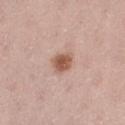notes: no biopsy performed (imaged during a skin exam); size: ~2.5 mm (longest diameter); patient: female, in their 40s; acquisition: 15 mm crop, total-body photography; tile lighting: white-light; anatomic site: the left thigh.